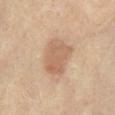Impression:
This lesion was catalogued during total-body skin photography and was not selected for biopsy.
Context:
A female subject, aged 63 to 67. A roughly 15 mm field-of-view crop from a total-body skin photograph. The lesion is on the leg.